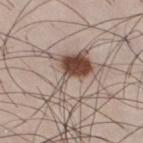biopsy status: no biopsy performed (imaged during a skin exam) | body site: the leg | imaging modality: 15 mm crop, total-body photography | tile lighting: white-light | subject: male, aged 33–37 | size: about 7.5 mm.